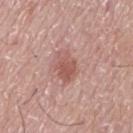This lesion was catalogued during total-body skin photography and was not selected for biopsy. This is a white-light tile. The lesion-visualizer software estimated a mean CIELAB color near L≈55 a*≈24 b*≈25, about 10 CIELAB-L* units darker than the surrounding skin, and a normalized lesion–skin contrast near 6.5. It also reported a classifier nevus-likeness of about 30/100 and a lesion-detection confidence of about 100/100. From the mid back. A male patient aged around 75. A 15 mm close-up extracted from a 3D total-body photography capture.